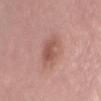Part of a total-body skin-imaging series; this lesion was reviewed on a skin check and was not flagged for biopsy. The total-body-photography lesion software estimated border irregularity of about 3.5 on a 0–10 scale and a color-variation rating of about 2/10. Captured under white-light illumination. A female patient, in their mid-30s. On the back. Cropped from a whole-body photographic skin survey; the tile spans about 15 mm. Approximately 3.5 mm at its widest.